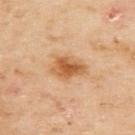Part of a total-body skin-imaging series; this lesion was reviewed on a skin check and was not flagged for biopsy.
Located on the back.
The lesion's longest dimension is about 4 mm.
A female patient in their 60s.
A close-up tile cropped from a whole-body skin photograph, about 15 mm across.